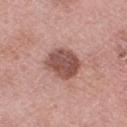Q: Is there a histopathology result?
A: no biopsy performed (imaged during a skin exam)
Q: Who is the patient?
A: female, aged approximately 70
Q: Lesion size?
A: about 4.5 mm
Q: How was this image acquired?
A: ~15 mm tile from a whole-body skin photo
Q: What did automated image analysis measure?
A: a classifier nevus-likeness of about 15/100 and a detector confidence of about 100 out of 100 that the crop contains a lesion
Q: Illumination type?
A: white-light illumination
Q: Lesion location?
A: the right thigh